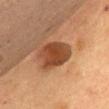Findings:
• follow-up — imaged on a skin check; not biopsied
• anatomic site — the chest
• diameter — ~5 mm (longest diameter)
• acquisition — ~15 mm tile from a whole-body skin photo
• automated metrics — a lesion area of about 13 mm² and a shape-asymmetry score of about 0.2 (0 = symmetric)
• patient — female, roughly 60 years of age